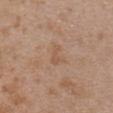Notes:
* notes — no biopsy performed (imaged during a skin exam)